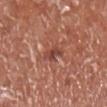workup: imaged on a skin check; not biopsied | patient: male, aged approximately 70 | body site: the right lower leg | size: about 2.5 mm | image: ~15 mm crop, total-body skin-cancer survey | tile lighting: white-light illumination.